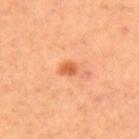Clinical impression: Captured during whole-body skin photography for melanoma surveillance; the lesion was not biopsied. Acquisition and patient details: The recorded lesion diameter is about 2 mm. The lesion is located on the back. The patient is a female in their 40s. Cropped from a whole-body photographic skin survey; the tile spans about 15 mm.